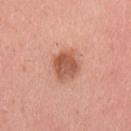The lesion was photographed on a routine skin check and not biopsied; there is no pathology result.
Cropped from a total-body skin-imaging series; the visible field is about 15 mm.
A female patient, about 25 years old.
Measured at roughly 3.5 mm in maximum diameter.
Captured under white-light illumination.
Automated image analysis of the tile measured a lesion area of about 9.5 mm², an eccentricity of roughly 0.5, and a shape-asymmetry score of about 0.15 (0 = symmetric). And it measured an automated nevus-likeness rating near 75 out of 100.
On the left upper arm.